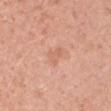Assessment: No biopsy was performed on this lesion — it was imaged during a full skin examination and was not determined to be concerning. Image and clinical context: The lesion is located on the left upper arm. A male patient roughly 45 years of age. About 2.5 mm across. Cropped from a total-body skin-imaging series; the visible field is about 15 mm.